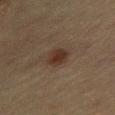Imaged during a routine full-body skin examination; the lesion was not biopsied and no histopathology is available. A female subject about 65 years old. The lesion-visualizer software estimated an area of roughly 5.5 mm² and a shape-asymmetry score of about 0.15 (0 = symmetric). It also reported a lesion–skin lightness drop of about 8 and a normalized border contrast of about 8.5. And it measured border irregularity of about 1.5 on a 0–10 scale, a within-lesion color-variation index near 2.5/10, and peripheral color asymmetry of about 0.5. The software also gave lesion-presence confidence of about 100/100. Cropped from a total-body skin-imaging series; the visible field is about 15 mm. Imaged with cross-polarized lighting. The lesion is on the front of the torso. The recorded lesion diameter is about 3 mm.